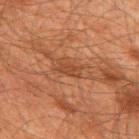The lesion was tiled from a total-body skin photograph and was not biopsied.
The subject is a male aged 58 to 62.
Automated image analysis of the tile measured an outline eccentricity of about 0.85 (0 = round, 1 = elongated) and two-axis asymmetry of about 0.2. And it measured a lesion color around L≈35 a*≈20 b*≈29 in CIELAB, a lesion–skin lightness drop of about 6, and a normalized border contrast of about 6. And it measured a color-variation rating of about 1.5/10 and peripheral color asymmetry of about 0.5.
About 3 mm across.
A region of skin cropped from a whole-body photographic capture, roughly 15 mm wide.
On the back.